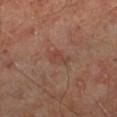Assessment: Part of a total-body skin-imaging series; this lesion was reviewed on a skin check and was not flagged for biopsy. Background: A male patient, aged 48–52. The lesion is on the left leg. Approximately 3.5 mm at its widest. Cropped from a total-body skin-imaging series; the visible field is about 15 mm.